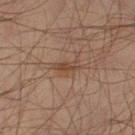No biopsy was performed on this lesion — it was imaged during a full skin examination and was not determined to be concerning. This image is a 15 mm lesion crop taken from a total-body photograph. The lesion is on the left lower leg. About 3.5 mm across. Automated image analysis of the tile measured a footprint of about 4.5 mm², a shape eccentricity near 0.85, and a symmetry-axis asymmetry near 0.3. The analysis additionally found a lesion color around L≈45 a*≈17 b*≈28 in CIELAB and a lesion–skin lightness drop of about 7. The software also gave a border-irregularity rating of about 3.5/10, a color-variation rating of about 3/10, and peripheral color asymmetry of about 1. The subject is a male aged 43–47.